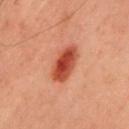{"biopsy_status": "not biopsied; imaged during a skin examination", "lighting": "cross-polarized", "patient": {"sex": "male", "age_approx": 40}, "site": "front of the torso", "lesion_size": {"long_diameter_mm_approx": 5.0}, "image": {"source": "total-body photography crop", "field_of_view_mm": 15}}